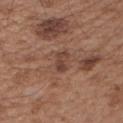Impression: This lesion was catalogued during total-body skin photography and was not selected for biopsy. Acquisition and patient details: An algorithmic analysis of the crop reported border irregularity of about 3 on a 0–10 scale and a within-lesion color-variation index near 2.5/10. And it measured a nevus-likeness score of about 0/100 and a lesion-detection confidence of about 100/100. The tile uses white-light illumination. About 3 mm across. Cropped from a total-body skin-imaging series; the visible field is about 15 mm. The lesion is on the left upper arm. A male subject aged 53–57.